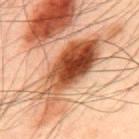Impression:
The lesion was tiled from a total-body skin photograph and was not biopsied.
Clinical summary:
Automated tile analysis of the lesion measured about 21 CIELAB-L* units darker than the surrounding skin and a normalized lesion–skin contrast near 13. The software also gave a border-irregularity index near 5/10, a within-lesion color-variation index near 10/10, and peripheral color asymmetry of about 5. Measured at roughly 11 mm in maximum diameter. The subject is a male roughly 50 years of age. On the back. Cropped from a total-body skin-imaging series; the visible field is about 15 mm.